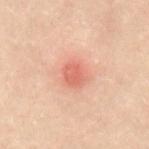Captured during whole-body skin photography for melanoma surveillance; the lesion was not biopsied.
A 15 mm close-up extracted from a 3D total-body photography capture.
The total-body-photography lesion software estimated a lesion area of about 5.5 mm², a shape eccentricity near 0.15, and a symmetry-axis asymmetry near 0.2.
The lesion is on the back.
The subject is a female in their mid- to late 50s.
The tile uses cross-polarized illumination.